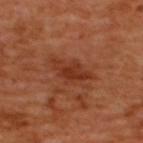No biopsy was performed on this lesion — it was imaged during a full skin examination and was not determined to be concerning. A 15 mm close-up extracted from a 3D total-body photography capture. A male patient, aged 48 to 52. Approximately 5 mm at its widest. Located on the upper back. This is a cross-polarized tile.